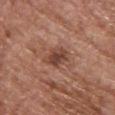lesion diameter — about 4 mm; acquisition — total-body-photography crop, ~15 mm field of view; lighting — white-light illumination; patient — female, aged 63–67; body site — the front of the torso.